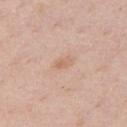Captured during whole-body skin photography for melanoma surveillance; the lesion was not biopsied.
Approximately 2.5 mm at its widest.
A 15 mm close-up tile from a total-body photography series done for melanoma screening.
The subject is a male aged 48 to 52.
This is a white-light tile.
The lesion is located on the chest.
Automated tile analysis of the lesion measured a border-irregularity index near 2.5/10 and a within-lesion color-variation index near 2.5/10.